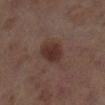| field | value |
|---|---|
| notes | catalogued during a skin exam; not biopsied |
| tile lighting | cross-polarized illumination |
| anatomic site | the right lower leg |
| acquisition | total-body-photography crop, ~15 mm field of view |
| image-analysis metrics | a classifier nevus-likeness of about 85/100 |
| subject | female, roughly 60 years of age |
| lesion diameter | ~4 mm (longest diameter) |